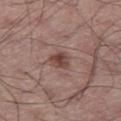follow-up: catalogued during a skin exam; not biopsied | tile lighting: white-light | automated metrics: a lesion area of about 4.5 mm², an eccentricity of roughly 0.55, and a symmetry-axis asymmetry near 0.3; a lesion–skin lightness drop of about 11 and a normalized border contrast of about 8.5; a nevus-likeness score of about 90/100 and a detector confidence of about 100 out of 100 that the crop contains a lesion | diameter: ~2.5 mm (longest diameter) | body site: the right thigh | subject: male, approximately 55 years of age | image: 15 mm crop, total-body photography.